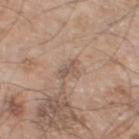Impression: The lesion was photographed on a routine skin check and not biopsied; there is no pathology result. Context: On the left lower leg. A region of skin cropped from a whole-body photographic capture, roughly 15 mm wide. The subject is a male roughly 60 years of age.